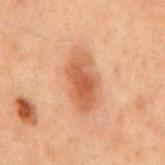workup = catalogued during a skin exam; not biopsied
location = the mid back
patient = male, in their 70s
image source = total-body-photography crop, ~15 mm field of view